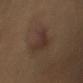lesion diameter: ~4 mm (longest diameter)
patient: male, aged around 65
image: ~15 mm crop, total-body skin-cancer survey
lighting: cross-polarized illumination
location: the left upper arm
image-analysis metrics: a footprint of about 7 mm², a shape eccentricity near 0.75, and a shape-asymmetry score of about 0.5 (0 = symmetric); a border-irregularity rating of about 5/10, internal color variation of about 2.5 on a 0–10 scale, and peripheral color asymmetry of about 1